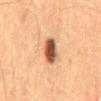Q: Was a biopsy performed?
A: total-body-photography surveillance lesion; no biopsy
Q: Patient demographics?
A: male, in their 60s
Q: How large is the lesion?
A: ~3.5 mm (longest diameter)
Q: What is the imaging modality?
A: ~15 mm crop, total-body skin-cancer survey